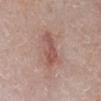Q: Was a biopsy performed?
A: catalogued during a skin exam; not biopsied
Q: Who is the patient?
A: male, approximately 65 years of age
Q: What kind of image is this?
A: 15 mm crop, total-body photography
Q: Lesion location?
A: the right lower leg